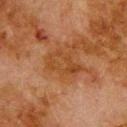Image and clinical context:
A male subject approximately 80 years of age. A lesion tile, about 15 mm wide, cut from a 3D total-body photograph. Located on the back.